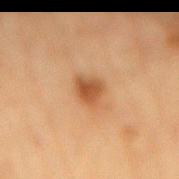Case summary:
- notes · catalogued during a skin exam; not biopsied
- patient · female, aged approximately 80
- size · about 2.5 mm
- lighting · cross-polarized
- location · the mid back
- imaging modality · ~15 mm crop, total-body skin-cancer survey